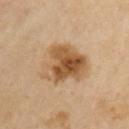Q: Was this lesion biopsied?
A: imaged on a skin check; not biopsied
Q: What is the anatomic site?
A: the chest
Q: What is the imaging modality?
A: 15 mm crop, total-body photography
Q: What is the lesion's diameter?
A: ~6 mm (longest diameter)
Q: Patient demographics?
A: male, aged 68 to 72